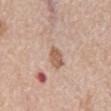  biopsy_status: not biopsied; imaged during a skin examination
  automated_metrics:
    area_mm2_approx: 7.5
    eccentricity: 0.8
    shape_asymmetry: 0.4
    nevus_likeness_0_100: 0
    lesion_detection_confidence_0_100: 100
  patient:
    sex: male
    age_approx: 65
  site: mid back
  lighting: white-light
  image:
    source: total-body photography crop
    field_of_view_mm: 15
  lesion_size:
    long_diameter_mm_approx: 4.0